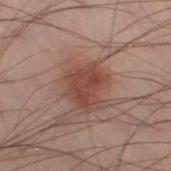No biopsy was performed on this lesion — it was imaged during a full skin examination and was not determined to be concerning. Captured under cross-polarized illumination. From the right thigh. Cropped from a total-body skin-imaging series; the visible field is about 15 mm. A male patient aged 38–42.